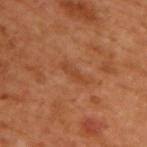<tbp_lesion>
  <biopsy_status>not biopsied; imaged during a skin examination</biopsy_status>
  <image>
    <source>total-body photography crop</source>
    <field_of_view_mm>15</field_of_view_mm>
  </image>
  <patient>
    <sex>male</sex>
    <age_approx>50</age_approx>
  </patient>
  <site>upper back</site>
</tbp_lesion>